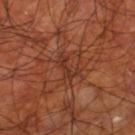{
  "biopsy_status": "not biopsied; imaged during a skin examination",
  "image": {
    "source": "total-body photography crop",
    "field_of_view_mm": 15
  },
  "site": "left thigh",
  "lesion_size": {
    "long_diameter_mm_approx": 3.5
  },
  "patient": {
    "sex": "male",
    "age_approx": 70
  },
  "lighting": "cross-polarized"
}